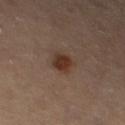  biopsy_status: not biopsied; imaged during a skin examination
  patient:
    sex: male
    age_approx: 85+
  site: left thigh
  image:
    source: total-body photography crop
    field_of_view_mm: 15